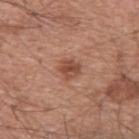Captured during whole-body skin photography for melanoma surveillance; the lesion was not biopsied.
The lesion is on the upper back.
A 15 mm crop from a total-body photograph taken for skin-cancer surveillance.
Imaged with white-light lighting.
The lesion-visualizer software estimated about 10 CIELAB-L* units darker than the surrounding skin and a lesion-to-skin contrast of about 7.5 (normalized; higher = more distinct). The software also gave a border-irregularity index near 2.5/10, internal color variation of about 3.5 on a 0–10 scale, and a peripheral color-asymmetry measure near 1.
A male patient, approximately 55 years of age.